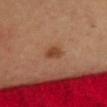Assessment: Imaged during a routine full-body skin examination; the lesion was not biopsied and no histopathology is available. Context: Cropped from a total-body skin-imaging series; the visible field is about 15 mm. The subject is a female aged 33 to 37. Located on the chest.